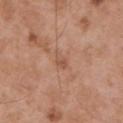biopsy status: imaged on a skin check; not biopsied
image source: 15 mm crop, total-body photography
illumination: white-light
subject: male, aged around 55
body site: the upper back
TBP lesion metrics: a lesion area of about 5 mm², a shape eccentricity near 0.8, and two-axis asymmetry of about 0.3; a within-lesion color-variation index near 3/10 and peripheral color asymmetry of about 1; a nevus-likeness score of about 0/100 and lesion-presence confidence of about 100/100